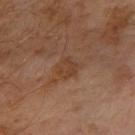Notes:
– follow-up · total-body-photography surveillance lesion; no biopsy
– patient · male, aged around 60
– automated lesion analysis · a border-irregularity index near 2/10 and a color-variation rating of about 1.5/10; a nevus-likeness score of about 0/100 and a detector confidence of about 100 out of 100 that the crop contains a lesion
– tile lighting · cross-polarized illumination
– body site · the arm
– acquisition · ~15 mm crop, total-body skin-cancer survey